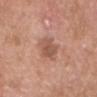The lesion was tiled from a total-body skin photograph and was not biopsied. This image is a 15 mm lesion crop taken from a total-body photograph. Longest diameter approximately 3.5 mm. The subject is a male in their mid- to late 60s. On the head or neck.